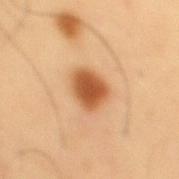Impression: This lesion was catalogued during total-body skin photography and was not selected for biopsy. Image and clinical context: A male patient, aged around 55. Imaged with cross-polarized lighting. Measured at roughly 3.5 mm in maximum diameter. The lesion is located on the mid back. A 15 mm close-up extracted from a 3D total-body photography capture. The lesion-visualizer software estimated a border-irregularity rating of about 2/10 and a within-lesion color-variation index near 3/10. It also reported a nevus-likeness score of about 100/100 and lesion-presence confidence of about 100/100.